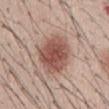follow-up: no biopsy performed (imaged during a skin exam)
size: about 6 mm
patient: male, approximately 40 years of age
tile lighting: white-light
automated metrics: an area of roughly 17 mm² and an outline eccentricity of about 0.7 (0 = round, 1 = elongated); an average lesion color of about L≈52 a*≈22 b*≈25 (CIELAB), about 14 CIELAB-L* units darker than the surrounding skin, and a lesion-to-skin contrast of about 9.5 (normalized; higher = more distinct)
acquisition: ~15 mm tile from a whole-body skin photo
location: the abdomen